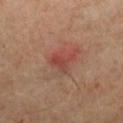workup: imaged on a skin check; not biopsied
lesion diameter: ~3 mm (longest diameter)
lighting: cross-polarized illumination
automated metrics: an area of roughly 5 mm², a shape eccentricity near 0.6, and a shape-asymmetry score of about 0.45 (0 = symmetric); a lesion–skin lightness drop of about 7 and a normalized border contrast of about 6; a within-lesion color-variation index near 2/10 and a peripheral color-asymmetry measure near 0.5
patient: male, in their mid- to late 50s
imaging modality: ~15 mm crop, total-body skin-cancer survey
site: the left lower leg